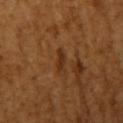<record>
  <site>left upper arm</site>
  <image>
    <source>total-body photography crop</source>
    <field_of_view_mm>15</field_of_view_mm>
  </image>
  <patient>
    <sex>female</sex>
    <age_approx>55</age_approx>
  </patient>
  <lighting>cross-polarized</lighting>
</record>